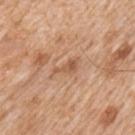Q: What is the anatomic site?
A: the left upper arm
Q: What is the imaging modality?
A: 15 mm crop, total-body photography
Q: Automated lesion metrics?
A: an average lesion color of about L≈56 a*≈22 b*≈35 (CIELAB), about 9 CIELAB-L* units darker than the surrounding skin, and a normalized lesion–skin contrast near 6.5; border irregularity of about 5.5 on a 0–10 scale, a within-lesion color-variation index near 1.5/10, and radial color variation of about 0.5; an automated nevus-likeness rating near 0 out of 100
Q: Patient demographics?
A: male, aged approximately 65